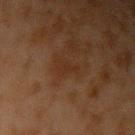Impression:
The lesion was tiled from a total-body skin photograph and was not biopsied.
Context:
Automated tile analysis of the lesion measured a footprint of about 3 mm² and a symmetry-axis asymmetry near 0.5. And it measured a mean CIELAB color near L≈24 a*≈16 b*≈25 and a normalized lesion–skin contrast near 5. The tile uses cross-polarized illumination. Cropped from a whole-body photographic skin survey; the tile spans about 15 mm. The lesion is on the arm. Measured at roughly 3 mm in maximum diameter. The patient is a male aged 43 to 47.